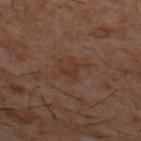Notes:
• workup · catalogued during a skin exam; not biopsied
• TBP lesion metrics · a lesion color around L≈31 a*≈17 b*≈24 in CIELAB, about 4 CIELAB-L* units darker than the surrounding skin, and a normalized lesion–skin contrast near 5.5
• subject · male, aged 58 to 62
• body site · the upper back
• imaging modality · ~15 mm tile from a whole-body skin photo